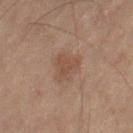notes: imaged on a skin check; not biopsied | lesion size: about 3.5 mm | imaging modality: total-body-photography crop, ~15 mm field of view | automated metrics: a footprint of about 8.5 mm² and an outline eccentricity of about 0.5 (0 = round, 1 = elongated); a mean CIELAB color near L≈36 a*≈14 b*≈22 and about 5 CIELAB-L* units darker than the surrounding skin | tile lighting: cross-polarized illumination | subject: male, approximately 70 years of age | anatomic site: the right thigh.